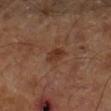No biopsy was performed on this lesion — it was imaged during a full skin examination and was not determined to be concerning.